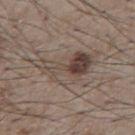{
  "biopsy_status": "not biopsied; imaged during a skin examination",
  "site": "chest",
  "image": {
    "source": "total-body photography crop",
    "field_of_view_mm": 15
  },
  "lesion_size": {
    "long_diameter_mm_approx": 7.5
  },
  "patient": {
    "sex": "male",
    "age_approx": 75
  }
}